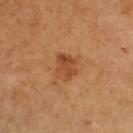lesion diameter=≈3.5 mm
TBP lesion metrics=a lesion area of about 6.5 mm² and two-axis asymmetry of about 0.25; a lesion–skin lightness drop of about 9 and a normalized lesion–skin contrast near 7; a nevus-likeness score of about 70/100
illumination=cross-polarized
subject=female, aged 53–57
image=~15 mm tile from a whole-body skin photo
body site=the right lower leg